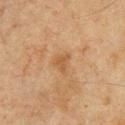Q: Is there a histopathology result?
A: total-body-photography surveillance lesion; no biopsy
Q: How was the tile lit?
A: cross-polarized illumination
Q: What are the patient's age and sex?
A: male, approximately 65 years of age
Q: What kind of image is this?
A: 15 mm crop, total-body photography
Q: Lesion location?
A: the chest